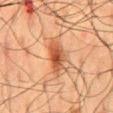The lesion was tiled from a total-body skin photograph and was not biopsied.
Captured under cross-polarized illumination.
From the mid back.
The lesion's longest dimension is about 4 mm.
A male subject, approximately 60 years of age.
This image is a 15 mm lesion crop taken from a total-body photograph.